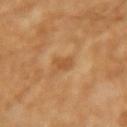Located on the right forearm. This image is a 15 mm lesion crop taken from a total-body photograph. The total-body-photography lesion software estimated a footprint of about 2.5 mm², an eccentricity of roughly 0.85, and a shape-asymmetry score of about 0.3 (0 = symmetric). The software also gave an average lesion color of about L≈54 a*≈22 b*≈41 (CIELAB), roughly 8 lightness units darker than nearby skin, and a lesion-to-skin contrast of about 6 (normalized; higher = more distinct). And it measured a border-irregularity index near 3/10, internal color variation of about 1 on a 0–10 scale, and radial color variation of about 0.5. It also reported a nevus-likeness score of about 0/100 and a lesion-detection confidence of about 100/100. Longest diameter approximately 2.5 mm. The tile uses cross-polarized illumination. The patient is a female aged around 70.